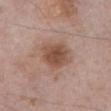Recorded during total-body skin imaging; not selected for excision or biopsy.
The tile uses white-light illumination.
The lesion-visualizer software estimated a lesion area of about 10 mm², a shape eccentricity near 0.35, and a shape-asymmetry score of about 0.2 (0 = symmetric). The analysis additionally found a border-irregularity rating of about 2/10 and radial color variation of about 1. The software also gave an automated nevus-likeness rating near 50 out of 100 and lesion-presence confidence of about 100/100.
The patient is a male in their mid-70s.
The lesion is on the abdomen.
Approximately 3.5 mm at its widest.
A region of skin cropped from a whole-body photographic capture, roughly 15 mm wide.